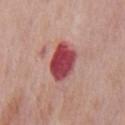biopsy_status: not biopsied; imaged during a skin examination
patient:
  sex: male
  age_approx: 75
lesion_size:
  long_diameter_mm_approx: 4.0
image:
  source: total-body photography crop
  field_of_view_mm: 15
lighting: white-light
site: mid back